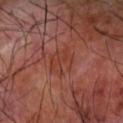The lesion was photographed on a routine skin check and not biopsied; there is no pathology result.
Located on the right forearm.
Captured under cross-polarized illumination.
A 15 mm crop from a total-body photograph taken for skin-cancer surveillance.
A male subject, aged around 70.